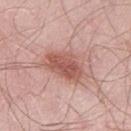biopsy_status: not biopsied; imaged during a skin examination
image:
  source: total-body photography crop
  field_of_view_mm: 15
site: left thigh
patient:
  sex: male
  age_approx: 55